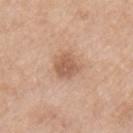The lesion was photographed on a routine skin check and not biopsied; there is no pathology result.
Cropped from a whole-body photographic skin survey; the tile spans about 15 mm.
A female subject aged 53–57.
From the left upper arm.